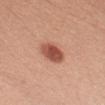Q: Was a biopsy performed?
A: imaged on a skin check; not biopsied
Q: Lesion location?
A: the left upper arm
Q: Patient demographics?
A: male, aged 28 to 32
Q: Automated lesion metrics?
A: a lesion color around L≈52 a*≈27 b*≈31 in CIELAB and a lesion-to-skin contrast of about 10 (normalized; higher = more distinct); peripheral color asymmetry of about 1; a classifier nevus-likeness of about 100/100 and a lesion-detection confidence of about 100/100
Q: What is the lesion's diameter?
A: ≈4.5 mm
Q: What is the imaging modality?
A: 15 mm crop, total-body photography